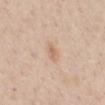Background:
Measured at roughly 3 mm in maximum diameter. The subject is a female approximately 45 years of age. The tile uses white-light illumination. A 15 mm crop from a total-body photograph taken for skin-cancer surveillance. The total-body-photography lesion software estimated an area of roughly 5 mm², an eccentricity of roughly 0.75, and two-axis asymmetry of about 0.2. The analysis additionally found a mean CIELAB color near L≈67 a*≈17 b*≈30 and roughly 7 lightness units darker than nearby skin. The lesion is located on the mid back.